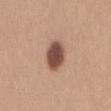No biopsy was performed on this lesion — it was imaged during a full skin examination and was not determined to be concerning.